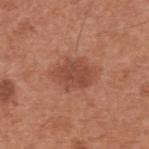Notes:
- tile lighting — white-light
- size — ≈5 mm
- anatomic site — the upper back
- patient — male, aged 53 to 57
- imaging modality — 15 mm crop, total-body photography